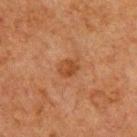| key | value |
|---|---|
| workup | imaged on a skin check; not biopsied |
| subject | male, about 60 years old |
| location | the upper back |
| image | total-body-photography crop, ~15 mm field of view |